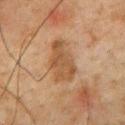Q: Was this lesion biopsied?
A: no biopsy performed (imaged during a skin exam)
Q: What lighting was used for the tile?
A: cross-polarized
Q: Where on the body is the lesion?
A: the chest
Q: Patient demographics?
A: male, aged 58–62
Q: What kind of image is this?
A: ~15 mm tile from a whole-body skin photo